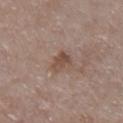Q: Is there a histopathology result?
A: total-body-photography surveillance lesion; no biopsy
Q: What kind of image is this?
A: total-body-photography crop, ~15 mm field of view
Q: What lighting was used for the tile?
A: white-light illumination
Q: Lesion size?
A: ~3 mm (longest diameter)
Q: What are the patient's age and sex?
A: female, roughly 85 years of age or older
Q: What is the anatomic site?
A: the chest